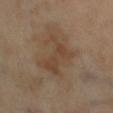Q: Was a biopsy performed?
A: catalogued during a skin exam; not biopsied
Q: Lesion location?
A: the right lower leg
Q: What lighting was used for the tile?
A: cross-polarized
Q: How was this image acquired?
A: total-body-photography crop, ~15 mm field of view
Q: Who is the patient?
A: female, approximately 70 years of age
Q: What is the lesion's diameter?
A: ~5 mm (longest diameter)